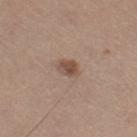No biopsy was performed on this lesion — it was imaged during a full skin examination and was not determined to be concerning. Cropped from a whole-body photographic skin survey; the tile spans about 15 mm. A female patient in their 40s. From the right thigh. An algorithmic analysis of the crop reported a footprint of about 4 mm², an eccentricity of roughly 0.6, and two-axis asymmetry of about 0.2. It also reported a detector confidence of about 100 out of 100 that the crop contains a lesion. The tile uses white-light illumination. Measured at roughly 2.5 mm in maximum diameter.